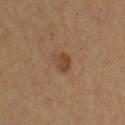Q: Is there a histopathology result?
A: imaged on a skin check; not biopsied
Q: What are the patient's age and sex?
A: female, approximately 60 years of age
Q: How was this image acquired?
A: ~15 mm crop, total-body skin-cancer survey
Q: Lesion location?
A: the right upper arm